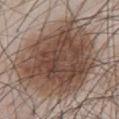| key | value |
|---|---|
| follow-up | imaged on a skin check; not biopsied |
| image-analysis metrics | a shape-asymmetry score of about 0.2 (0 = symmetric); a lesion-to-skin contrast of about 10.5 (normalized; higher = more distinct); a classifier nevus-likeness of about 80/100 and a lesion-detection confidence of about 100/100 |
| subject | male, about 55 years old |
| body site | the abdomen |
| image source | ~15 mm tile from a whole-body skin photo |
| lesion size | ≈11.5 mm |
| tile lighting | white-light illumination |